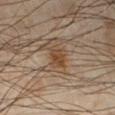notes — no biopsy performed (imaged during a skin exam) | subject — male, aged approximately 40 | body site — the right lower leg | image — 15 mm crop, total-body photography | size — ~2 mm (longest diameter).